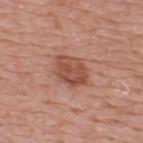Recorded during total-body skin imaging; not selected for excision or biopsy. A male patient, about 75 years old. On the upper back. The tile uses white-light illumination. Cropped from a whole-body photographic skin survey; the tile spans about 15 mm.